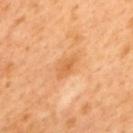<record>
  <biopsy_status>not biopsied; imaged during a skin examination</biopsy_status>
</record>